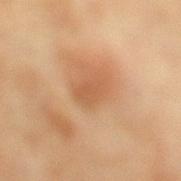From the leg. Cropped from a whole-body photographic skin survey; the tile spans about 15 mm. Captured under cross-polarized illumination. Measured at roughly 3.5 mm in maximum diameter. A female patient aged approximately 55.